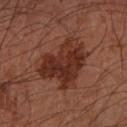{"biopsy_status": "not biopsied; imaged during a skin examination", "image": {"source": "total-body photography crop", "field_of_view_mm": 15}, "lighting": "cross-polarized", "automated_metrics": {"border_irregularity_0_10": 5.0, "color_variation_0_10": 4.5, "peripheral_color_asymmetry": 1.5, "nevus_likeness_0_100": 60}, "site": "left forearm", "patient": {"sex": "male", "age_approx": 65}}